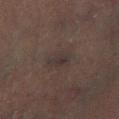Assessment:
The lesion was tiled from a total-body skin photograph and was not biopsied.
Acquisition and patient details:
A male subject aged 58 to 62. This is a cross-polarized tile. Measured at roughly 4 mm in maximum diameter. A roughly 15 mm field-of-view crop from a total-body skin photograph.